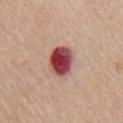| key | value |
|---|---|
| notes | imaged on a skin check; not biopsied |
| lesion diameter | ~4 mm (longest diameter) |
| image-analysis metrics | an average lesion color of about L≈46 a*≈33 b*≈22 (CIELAB), about 21 CIELAB-L* units darker than the surrounding skin, and a lesion-to-skin contrast of about 14.5 (normalized; higher = more distinct); a lesion-detection confidence of about 100/100 |
| subject | male, aged around 65 |
| location | the chest |
| tile lighting | white-light illumination |
| image source | 15 mm crop, total-body photography |